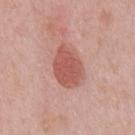Clinical impression: The lesion was photographed on a routine skin check and not biopsied; there is no pathology result. Image and clinical context: A male patient aged 48 to 52. Cropped from a whole-body photographic skin survey; the tile spans about 15 mm. The recorded lesion diameter is about 5 mm. This is a white-light tile. On the front of the torso.